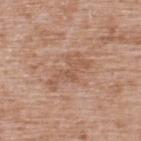Cropped from a total-body skin-imaging series; the visible field is about 15 mm. About 5 mm across. The lesion is located on the upper back. A male subject, aged around 50. The tile uses white-light illumination.